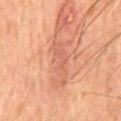Notes:
• workup — imaged on a skin check; not biopsied
• imaging modality — ~15 mm tile from a whole-body skin photo
• size — ≈5.5 mm
• patient — male, aged 63 to 67
• illumination — cross-polarized illumination
• body site — the mid back